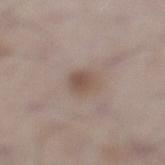Recorded during total-body skin imaging; not selected for excision or biopsy. Longest diameter approximately 3 mm. Located on the leg. The total-body-photography lesion software estimated a lesion area of about 5.5 mm², an outline eccentricity of about 0.7 (0 = round, 1 = elongated), and a shape-asymmetry score of about 0.15 (0 = symmetric). And it measured a color-variation rating of about 3/10 and radial color variation of about 1. And it measured a classifier nevus-likeness of about 80/100 and a detector confidence of about 100 out of 100 that the crop contains a lesion. A male subject, approximately 70 years of age. Captured under white-light illumination. Cropped from a whole-body photographic skin survey; the tile spans about 15 mm.